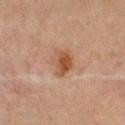| field | value |
|---|---|
| workup | no biopsy performed (imaged during a skin exam) |
| body site | the right thigh |
| patient | female, aged around 70 |
| image source | 15 mm crop, total-body photography |
| tile lighting | cross-polarized |
| diameter | ~3.5 mm (longest diameter) |